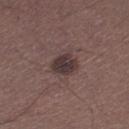Impression: Captured during whole-body skin photography for melanoma surveillance; the lesion was not biopsied. Acquisition and patient details: This is a white-light tile. On the right lower leg. Cropped from a whole-body photographic skin survey; the tile spans about 15 mm. The patient is a male in their 40s. Longest diameter approximately 3 mm.